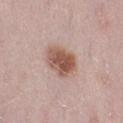{
  "biopsy_status": "not biopsied; imaged during a skin examination",
  "site": "left thigh",
  "patient": {
    "sex": "female",
    "age_approx": 30
  },
  "image": {
    "source": "total-body photography crop",
    "field_of_view_mm": 15
  }
}